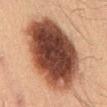biopsy status=no biopsy performed (imaged during a skin exam)
anatomic site=the abdomen
patient=male, approximately 55 years of age
lighting=cross-polarized illumination
acquisition=15 mm crop, total-body photography
automated metrics=a lesion color around L≈38 a*≈20 b*≈26 in CIELAB, about 21 CIELAB-L* units darker than the surrounding skin, and a normalized lesion–skin contrast near 16; a nevus-likeness score of about 60/100 and a detector confidence of about 100 out of 100 that the crop contains a lesion
lesion diameter=~10 mm (longest diameter)